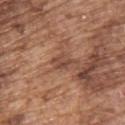Assessment: This lesion was catalogued during total-body skin photography and was not selected for biopsy. Acquisition and patient details: Captured under white-light illumination. Located on the back. A 15 mm crop from a total-body photograph taken for skin-cancer surveillance. Measured at roughly 2.5 mm in maximum diameter. Automated image analysis of the tile measured a footprint of about 3 mm², a shape eccentricity near 0.75, and two-axis asymmetry of about 0.45. The analysis additionally found a nevus-likeness score of about 0/100. A male subject in their mid- to late 70s.